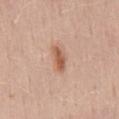No biopsy was performed on this lesion — it was imaged during a full skin examination and was not determined to be concerning. From the mid back. About 3.5 mm across. Automated tile analysis of the lesion measured a footprint of about 4.5 mm². The software also gave border irregularity of about 2.5 on a 0–10 scale, internal color variation of about 3 on a 0–10 scale, and radial color variation of about 1. The analysis additionally found an automated nevus-likeness rating near 100 out of 100 and lesion-presence confidence of about 100/100. A male subject aged 38 to 42. The tile uses white-light illumination. Cropped from a total-body skin-imaging series; the visible field is about 15 mm.